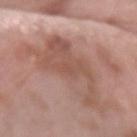Recorded during total-body skin imaging; not selected for excision or biopsy. A female patient, about 60 years old. A 15 mm crop from a total-body photograph taken for skin-cancer surveillance. Located on the arm.